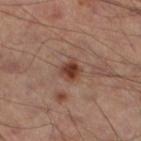Clinical impression: Part of a total-body skin-imaging series; this lesion was reviewed on a skin check and was not flagged for biopsy. Background: The subject is a male aged approximately 50. A region of skin cropped from a whole-body photographic capture, roughly 15 mm wide. Located on the left leg. An algorithmic analysis of the crop reported an automated nevus-likeness rating near 95 out of 100 and a lesion-detection confidence of about 100/100.